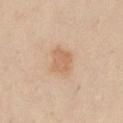This lesion was catalogued during total-body skin photography and was not selected for biopsy. On the chest. The tile uses white-light illumination. A 15 mm close-up extracted from a 3D total-body photography capture. The patient is a male roughly 35 years of age.